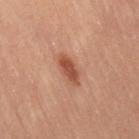Clinical impression:
Imaged during a routine full-body skin examination; the lesion was not biopsied and no histopathology is available.
Context:
About 4 mm across. Captured under cross-polarized illumination. The lesion is located on the right thigh. A 15 mm crop from a total-body photograph taken for skin-cancer surveillance. The total-body-photography lesion software estimated a footprint of about 6 mm², a shape eccentricity near 0.9, and a symmetry-axis asymmetry near 0.25. The analysis additionally found roughly 12 lightness units darker than nearby skin and a normalized border contrast of about 8.5. The subject is a female aged around 45.